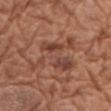Part of a total-body skin-imaging series; this lesion was reviewed on a skin check and was not flagged for biopsy. This is a white-light tile. A female patient aged around 75. The lesion is on the left upper arm. A close-up tile cropped from a whole-body skin photograph, about 15 mm across.